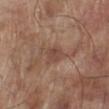{"biopsy_status": "not biopsied; imaged during a skin examination", "image": {"source": "total-body photography crop", "field_of_view_mm": 15}, "site": "left lower leg", "patient": {"sex": "male", "age_approx": 70}, "lighting": "white-light", "lesion_size": {"long_diameter_mm_approx": 2.5}}